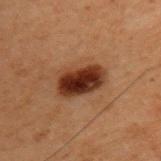follow-up: no biopsy performed (imaged during a skin exam) | patient: male, in their 60s | acquisition: 15 mm crop, total-body photography | site: the back.